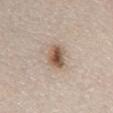Clinical summary:
A male patient aged around 80. On the abdomen. A 15 mm close-up extracted from a 3D total-body photography capture.